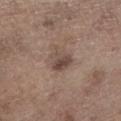The total-body-photography lesion software estimated border irregularity of about 3.5 on a 0–10 scale, internal color variation of about 5.5 on a 0–10 scale, and peripheral color asymmetry of about 2.5. Located on the left lower leg. A male patient roughly 70 years of age. This is a white-light tile. Cropped from a total-body skin-imaging series; the visible field is about 15 mm.